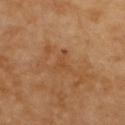• follow-up · imaged on a skin check; not biopsied
• patient · female, aged approximately 60
• diameter · ~2.5 mm (longest diameter)
• imaging modality · 15 mm crop, total-body photography
• lighting · cross-polarized illumination
• anatomic site · the upper back